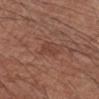No biopsy was performed on this lesion — it was imaged during a full skin examination and was not determined to be concerning.
Cropped from a total-body skin-imaging series; the visible field is about 15 mm.
From the right forearm.
The lesion's longest dimension is about 2.5 mm.
An algorithmic analysis of the crop reported an area of roughly 3.5 mm², a shape eccentricity near 0.7, and a symmetry-axis asymmetry near 0.35. The software also gave a mean CIELAB color near L≈41 a*≈23 b*≈26 and about 6 CIELAB-L* units darker than the surrounding skin. And it measured a border-irregularity index near 4/10, a color-variation rating of about 1.5/10, and a peripheral color-asymmetry measure near 0.5.
The tile uses white-light illumination.
A male patient, aged approximately 65.